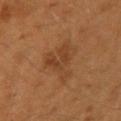follow-up: total-body-photography surveillance lesion; no biopsy | image-analysis metrics: an area of roughly 11 mm², an outline eccentricity of about 0.75 (0 = round, 1 = elongated), and a shape-asymmetry score of about 0.5 (0 = symmetric); a border-irregularity index near 6.5/10, internal color variation of about 3 on a 0–10 scale, and a peripheral color-asymmetry measure near 1; a classifier nevus-likeness of about 5/100 and a lesion-detection confidence of about 100/100 | location: the arm | image source: total-body-photography crop, ~15 mm field of view | size: about 5.5 mm | patient: male, aged 38–42.